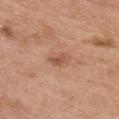Findings:
- workup — no biopsy performed (imaged during a skin exam)
- subject — female, aged around 65
- lesion diameter — ≈2.5 mm
- lighting — white-light
- anatomic site — the chest
- acquisition — ~15 mm tile from a whole-body skin photo